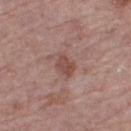biopsy status: catalogued during a skin exam; not biopsied
site: the right thigh
TBP lesion metrics: a mean CIELAB color near L≈48 a*≈21 b*≈24, a lesion–skin lightness drop of about 9, and a lesion-to-skin contrast of about 7 (normalized; higher = more distinct)
subject: female, aged around 65
acquisition: 15 mm crop, total-body photography
size: ≈3 mm